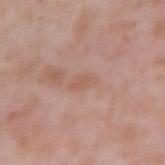<case>
  <lighting>white-light</lighting>
  <site>right upper arm</site>
  <image>
    <source>total-body photography crop</source>
    <field_of_view_mm>15</field_of_view_mm>
  </image>
  <patient>
    <sex>male</sex>
    <age_approx>55</age_approx>
  </patient>
  <lesion_size>
    <long_diameter_mm_approx>2.5</long_diameter_mm_approx>
  </lesion_size>
</case>